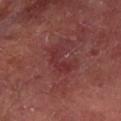Q: Was a biopsy performed?
A: total-body-photography surveillance lesion; no biopsy
Q: What are the patient's age and sex?
A: male, aged approximately 65
Q: What is the anatomic site?
A: the left forearm
Q: What did automated image analysis measure?
A: a footprint of about 6 mm² and a shape eccentricity near 0.85; a mean CIELAB color near L≈32 a*≈28 b*≈20, roughly 6 lightness units darker than nearby skin, and a normalized lesion–skin contrast near 5.5; a nevus-likeness score of about 0/100 and a detector confidence of about 95 out of 100 that the crop contains a lesion
Q: How large is the lesion?
A: ≈4 mm
Q: How was this image acquired?
A: 15 mm crop, total-body photography
Q: What lighting was used for the tile?
A: cross-polarized